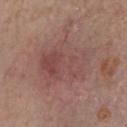Captured during whole-body skin photography for melanoma surveillance; the lesion was not biopsied. The lesion is on the front of the torso. An algorithmic analysis of the crop reported two-axis asymmetry of about 0.25. The analysis additionally found a nevus-likeness score of about 0/100 and a detector confidence of about 100 out of 100 that the crop contains a lesion. Cropped from a total-body skin-imaging series; the visible field is about 15 mm. A male patient approximately 70 years of age. The lesion's longest dimension is about 7.5 mm.